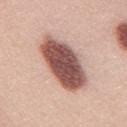notes: imaged on a skin check; not biopsied | location: the mid back | image: ~15 mm crop, total-body skin-cancer survey | lesion size: ~8 mm (longest diameter) | tile lighting: white-light illumination | automated lesion analysis: an area of roughly 25 mm², an outline eccentricity of about 0.9 (0 = round, 1 = elongated), and a shape-asymmetry score of about 0.15 (0 = symmetric); a lesion color around L≈53 a*≈22 b*≈24 in CIELAB and a normalized border contrast of about 13.5; a border-irregularity rating of about 2/10 and internal color variation of about 5 on a 0–10 scale | patient: female, aged 23 to 27.